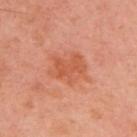This lesion was catalogued during total-body skin photography and was not selected for biopsy.
The lesion is located on the upper back.
The subject is a male aged 48–52.
This image is a 15 mm lesion crop taken from a total-body photograph.
The recorded lesion diameter is about 4.5 mm.
The tile uses cross-polarized illumination.